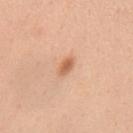Clinical impression:
Part of a total-body skin-imaging series; this lesion was reviewed on a skin check and was not flagged for biopsy.
Clinical summary:
Measured at roughly 3 mm in maximum diameter. The subject is a female about 35 years old. Imaged with white-light lighting. The lesion is on the upper back. A roughly 15 mm field-of-view crop from a total-body skin photograph.